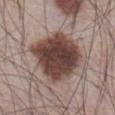No biopsy was performed on this lesion — it was imaged during a full skin examination and was not determined to be concerning. Automated image analysis of the tile measured a lesion area of about 32 mm², an outline eccentricity of about 0.25 (0 = round, 1 = elongated), and two-axis asymmetry of about 0.15. And it measured an average lesion color of about L≈42 a*≈17 b*≈21 (CIELAB), roughly 18 lightness units darker than nearby skin, and a lesion-to-skin contrast of about 13 (normalized; higher = more distinct). The analysis additionally found border irregularity of about 2 on a 0–10 scale, a within-lesion color-variation index near 5.5/10, and a peripheral color-asymmetry measure near 1.5. Captured under white-light illumination. From the abdomen. A male patient, about 65 years old. A roughly 15 mm field-of-view crop from a total-body skin photograph.